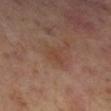This lesion was catalogued during total-body skin photography and was not selected for biopsy. On the right lower leg. A 15 mm close-up tile from a total-body photography series done for melanoma screening. The tile uses cross-polarized illumination. About 3 mm across. The subject is a female about 55 years old. An algorithmic analysis of the crop reported a border-irregularity index near 3.5/10, internal color variation of about 2 on a 0–10 scale, and radial color variation of about 0.5. It also reported lesion-presence confidence of about 100/100.